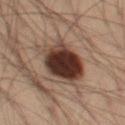Impression: Part of a total-body skin-imaging series; this lesion was reviewed on a skin check and was not flagged for biopsy. Image and clinical context: Automated image analysis of the tile measured a footprint of about 23 mm², an eccentricity of roughly 0.65, and a symmetry-axis asymmetry near 0.2. And it measured lesion-presence confidence of about 100/100. Imaged with cross-polarized lighting. From the left thigh. The lesion's longest dimension is about 6.5 mm. A male patient, approximately 55 years of age. A close-up tile cropped from a whole-body skin photograph, about 15 mm across.